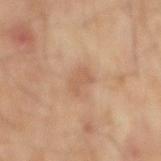Impression:
Captured during whole-body skin photography for melanoma surveillance; the lesion was not biopsied.